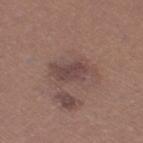Context: A close-up tile cropped from a whole-body skin photograph, about 15 mm across. The subject is a female approximately 40 years of age. The lesion is located on the leg. Captured under white-light illumination. The recorded lesion diameter is about 5 mm. An algorithmic analysis of the crop reported an area of roughly 8.5 mm² and two-axis asymmetry of about 0.35. The software also gave a mean CIELAB color near L≈44 a*≈18 b*≈19, about 8 CIELAB-L* units darker than the surrounding skin, and a normalized border contrast of about 7.5. It also reported a border-irregularity rating of about 4.5/10, a within-lesion color-variation index near 2/10, and peripheral color asymmetry of about 0.5. The analysis additionally found a classifier nevus-likeness of about 10/100 and lesion-presence confidence of about 100/100.